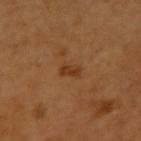Findings:
– illumination — cross-polarized
– anatomic site — the right upper arm
– patient — female, aged approximately 55
– diameter — about 2.5 mm
– acquisition — 15 mm crop, total-body photography